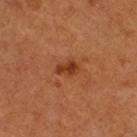notes: total-body-photography surveillance lesion; no biopsy
lesion diameter: about 2.5 mm
image-analysis metrics: an area of roughly 3.5 mm² and a shape-asymmetry score of about 0.25 (0 = symmetric); an average lesion color of about L≈38 a*≈29 b*≈37 (CIELAB), about 10 CIELAB-L* units darker than the surrounding skin, and a normalized lesion–skin contrast near 8.5; an automated nevus-likeness rating near 85 out of 100 and a detector confidence of about 100 out of 100 that the crop contains a lesion
subject: male, roughly 50 years of age
illumination: cross-polarized
image source: total-body-photography crop, ~15 mm field of view
site: the leg